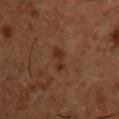The lesion was photographed on a routine skin check and not biopsied; there is no pathology result.
A roughly 15 mm field-of-view crop from a total-body skin photograph.
Located on the front of the torso.
The lesion-visualizer software estimated a classifier nevus-likeness of about 0/100 and a detector confidence of about 100 out of 100 that the crop contains a lesion.
Captured under cross-polarized illumination.
About 3 mm across.
The subject is a male aged around 55.